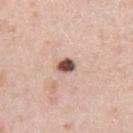biopsy status = no biopsy performed (imaged during a skin exam) | subject = male, aged 38–42 | image source = ~15 mm crop, total-body skin-cancer survey | lesion size = ≈2 mm | body site = the arm.